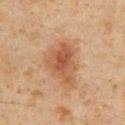| feature | finding |
|---|---|
| workup | total-body-photography surveillance lesion; no biopsy |
| diameter | about 5 mm |
| imaging modality | ~15 mm crop, total-body skin-cancer survey |
| image-analysis metrics | an area of roughly 13 mm², an eccentricity of roughly 0.7, and a symmetry-axis asymmetry near 0.35; roughly 9 lightness units darker than nearby skin and a normalized border contrast of about 7.5 |
| anatomic site | the chest |
| tile lighting | cross-polarized |
| patient | male, roughly 60 years of age |